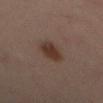workup: no biopsy performed (imaged during a skin exam) | patient: male, in their 50s | image-analysis metrics: a lesion–skin lightness drop of about 8 and a normalized border contrast of about 8.5; radial color variation of about 1 | site: the mid back | image source: ~15 mm tile from a whole-body skin photo | tile lighting: cross-polarized illumination.